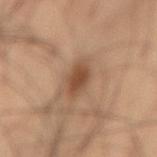Notes:
– biopsy status · catalogued during a skin exam; not biopsied
– site · the lower back
– subject · male, in their mid-50s
– image source · 15 mm crop, total-body photography
– illumination · cross-polarized